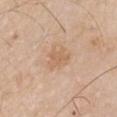Notes:
* biopsy status · imaged on a skin check; not biopsied
* TBP lesion metrics · a mean CIELAB color near L≈62 a*≈18 b*≈33, a lesion–skin lightness drop of about 7, and a normalized border contrast of about 5; a within-lesion color-variation index near 2/10 and peripheral color asymmetry of about 1; an automated nevus-likeness rating near 5 out of 100 and a lesion-detection confidence of about 100/100
* lesion diameter · about 3 mm
* subject · male, in their mid- to late 60s
* acquisition · ~15 mm tile from a whole-body skin photo
* body site · the right upper arm